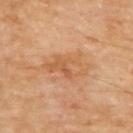• follow-up: total-body-photography surveillance lesion; no biopsy
• imaging modality: ~15 mm crop, total-body skin-cancer survey
• subject: male, aged 68 to 72
• site: the upper back